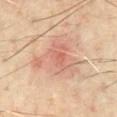workup: total-body-photography surveillance lesion; no biopsy
image-analysis metrics: a lesion area of about 21 mm², a shape eccentricity near 0.4, and two-axis asymmetry of about 0.55; a mean CIELAB color near L≈63 a*≈22 b*≈29 and a lesion–skin lightness drop of about 8; a border-irregularity rating of about 6/10 and peripheral color asymmetry of about 2
subject: male, approximately 60 years of age
image: ~15 mm crop, total-body skin-cancer survey
lesion diameter: ≈6.5 mm
site: the chest